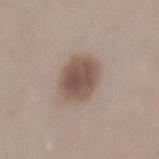Clinical impression:
Recorded during total-body skin imaging; not selected for excision or biopsy.
Image and clinical context:
This is a white-light tile. A male subject in their 30s. Cropped from a whole-body photographic skin survey; the tile spans about 15 mm. Located on the back.